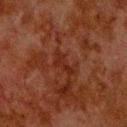Clinical summary:
This is a cross-polarized tile. A male subject about 80 years old. A roughly 15 mm field-of-view crop from a total-body skin photograph. The lesion is on the upper back. An algorithmic analysis of the crop reported a footprint of about 3.5 mm² and a symmetry-axis asymmetry near 0.6. The analysis additionally found a lesion-detection confidence of about 75/100.